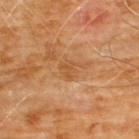{
  "biopsy_status": "not biopsied; imaged during a skin examination",
  "lighting": "cross-polarized",
  "image": {
    "source": "total-body photography crop",
    "field_of_view_mm": 15
  },
  "lesion_size": {
    "long_diameter_mm_approx": 3.0
  },
  "patient": {
    "sex": "male",
    "age_approx": 60
  },
  "site": "chest",
  "automated_metrics": {
    "shape_asymmetry": 0.45,
    "border_irregularity_0_10": 5.0,
    "color_variation_0_10": 2.0,
    "peripheral_color_asymmetry": 0.5
  }
}